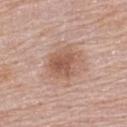{
  "biopsy_status": "not biopsied; imaged during a skin examination",
  "lighting": "white-light",
  "image": {
    "source": "total-body photography crop",
    "field_of_view_mm": 15
  },
  "site": "upper back",
  "patient": {
    "sex": "female",
    "age_approx": 65
  },
  "lesion_size": {
    "long_diameter_mm_approx": 4.0
  }
}